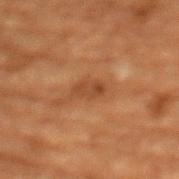Assessment:
Captured during whole-body skin photography for melanoma surveillance; the lesion was not biopsied.
Context:
The lesion is located on the chest. Longest diameter approximately 3 mm. The patient is a female about 80 years old. Cropped from a total-body skin-imaging series; the visible field is about 15 mm. The tile uses cross-polarized illumination.